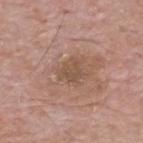workup = catalogued during a skin exam; not biopsied | imaging modality = ~15 mm tile from a whole-body skin photo | site = the upper back | patient = male, aged approximately 65 | lesion size = ~3 mm (longest diameter).